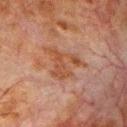Clinical impression: Captured during whole-body skin photography for melanoma surveillance; the lesion was not biopsied. Acquisition and patient details: About 6 mm across. Cropped from a whole-body photographic skin survey; the tile spans about 15 mm. This is a cross-polarized tile. A male patient about 80 years old. The lesion is located on the chest.